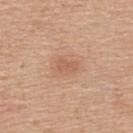Captured during whole-body skin photography for melanoma surveillance; the lesion was not biopsied. A 15 mm crop from a total-body photograph taken for skin-cancer surveillance. A male patient, aged 53–57. The tile uses white-light illumination. The lesion's longest dimension is about 2.5 mm. Located on the upper back.